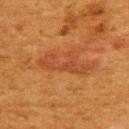This lesion was catalogued during total-body skin photography and was not selected for biopsy.
Automated image analysis of the tile measured an area of roughly 9.5 mm², an eccentricity of roughly 0.95, and a symmetry-axis asymmetry near 0.45. It also reported a lesion–skin lightness drop of about 6 and a lesion-to-skin contrast of about 6 (normalized; higher = more distinct). And it measured a color-variation rating of about 2/10 and peripheral color asymmetry of about 0.5. It also reported a nevus-likeness score of about 5/100 and a detector confidence of about 95 out of 100 that the crop contains a lesion.
Cropped from a whole-body photographic skin survey; the tile spans about 15 mm.
Located on the upper back.
A female patient aged 48–52.
About 6 mm across.